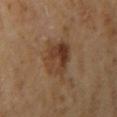Impression:
The lesion was tiled from a total-body skin photograph and was not biopsied.
Clinical summary:
The lesion's longest dimension is about 4.5 mm. Captured under cross-polarized illumination. A roughly 15 mm field-of-view crop from a total-body skin photograph. The subject is a female aged 58 to 62. The lesion is located on the arm.